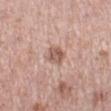{
  "biopsy_status": "not biopsied; imaged during a skin examination",
  "lighting": "white-light",
  "automated_metrics": {
    "border_irregularity_0_10": 2.0,
    "color_variation_0_10": 4.0,
    "peripheral_color_asymmetry": 1.5
  },
  "patient": {
    "sex": "female",
    "age_approx": 40
  },
  "lesion_size": {
    "long_diameter_mm_approx": 2.5
  },
  "image": {
    "source": "total-body photography crop",
    "field_of_view_mm": 15
  },
  "site": "right lower leg"
}